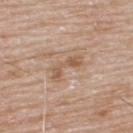The lesion is on the upper back. A lesion tile, about 15 mm wide, cut from a 3D total-body photograph. A male subject in their mid-60s.